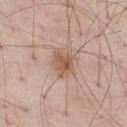{
  "biopsy_status": "not biopsied; imaged during a skin examination",
  "image": {
    "source": "total-body photography crop",
    "field_of_view_mm": 15
  },
  "lighting": "white-light",
  "patient": {
    "sex": "male",
    "age_approx": 55
  },
  "lesion_size": {
    "long_diameter_mm_approx": 3.0
  },
  "site": "right thigh"
}